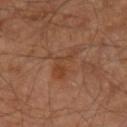biopsy status = total-body-photography surveillance lesion; no biopsy | body site = the right leg | lesion size = about 4.5 mm | image source = ~15 mm crop, total-body skin-cancer survey | patient = male, aged 58 to 62.